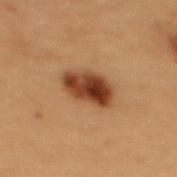Q: Is there a histopathology result?
A: total-body-photography surveillance lesion; no biopsy
Q: Automated lesion metrics?
A: a lesion color around L≈36 a*≈21 b*≈31 in CIELAB, a lesion–skin lightness drop of about 15, and a lesion-to-skin contrast of about 12 (normalized; higher = more distinct); a color-variation rating of about 7/10 and peripheral color asymmetry of about 2.5
Q: What is the imaging modality?
A: ~15 mm crop, total-body skin-cancer survey
Q: How was the tile lit?
A: cross-polarized
Q: What is the anatomic site?
A: the upper back
Q: Who is the patient?
A: female, approximately 50 years of age
Q: How large is the lesion?
A: about 4.5 mm